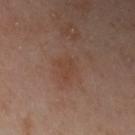The lesion was photographed on a routine skin check and not biopsied; there is no pathology result. A 15 mm close-up extracted from a 3D total-body photography capture. The lesion-visualizer software estimated a footprint of about 6 mm², a shape eccentricity near 0.7, and a shape-asymmetry score of about 0.35 (0 = symmetric). It also reported a mean CIELAB color near L≈42 a*≈19 b*≈27, a lesion–skin lightness drop of about 5, and a normalized lesion–skin contrast near 5. The software also gave border irregularity of about 4 on a 0–10 scale, a within-lesion color-variation index near 1.5/10, and a peripheral color-asymmetry measure near 0.5. Measured at roughly 3.5 mm in maximum diameter. A female subject, approximately 40 years of age. On the left upper arm.